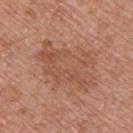biopsy_status: not biopsied; imaged during a skin examination
site: upper back
lesion_size:
  long_diameter_mm_approx: 7.5
patient:
  sex: male
  age_approx: 55
image:
  source: total-body photography crop
  field_of_view_mm: 15